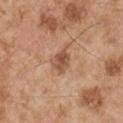<tbp_lesion>
<biopsy_status>not biopsied; imaged during a skin examination</biopsy_status>
<image>
  <source>total-body photography crop</source>
  <field_of_view_mm>15</field_of_view_mm>
</image>
<patient>
  <sex>male</sex>
  <age_approx>55</age_approx>
</patient>
<site>right upper arm</site>
</tbp_lesion>